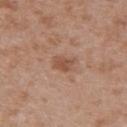From the upper back. A 15 mm close-up extracted from a 3D total-body photography capture. The subject is a female aged 28 to 32.